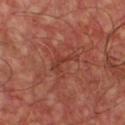No biopsy was performed on this lesion — it was imaged during a full skin examination and was not determined to be concerning. The recorded lesion diameter is about 3.5 mm. The subject is a male approximately 55 years of age. A close-up tile cropped from a whole-body skin photograph, about 15 mm across. On the chest.